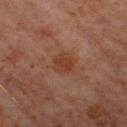Notes:
– subject — male, aged approximately 60
– anatomic site — the right thigh
– acquisition — total-body-photography crop, ~15 mm field of view
– tile lighting — cross-polarized illumination